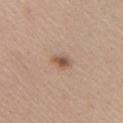The lesion was tiled from a total-body skin photograph and was not biopsied. Cropped from a whole-body photographic skin survey; the tile spans about 15 mm. Located on the right upper arm. A female patient, aged approximately 40. The total-body-photography lesion software estimated an automated nevus-likeness rating near 90 out of 100 and a detector confidence of about 100 out of 100 that the crop contains a lesion. This is a white-light tile. Longest diameter approximately 2.5 mm.